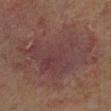Q: Was this lesion biopsied?
A: imaged on a skin check; not biopsied